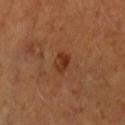{"biopsy_status": "not biopsied; imaged during a skin examination", "patient": {"sex": "female", "age_approx": 55}, "lighting": "cross-polarized", "site": "right forearm", "image": {"source": "total-body photography crop", "field_of_view_mm": 15}}